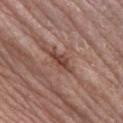Assessment:
Part of a total-body skin-imaging series; this lesion was reviewed on a skin check and was not flagged for biopsy.
Background:
Located on the right lower leg. A roughly 15 mm field-of-view crop from a total-body skin photograph. The lesion-visualizer software estimated a lesion area of about 4.5 mm² and a shape eccentricity near 0.9. It also reported a lesion–skin lightness drop of about 11 and a normalized lesion–skin contrast near 8.5. The software also gave a border-irregularity rating of about 3.5/10, internal color variation of about 3 on a 0–10 scale, and a peripheral color-asymmetry measure near 1. A male patient in their 70s.